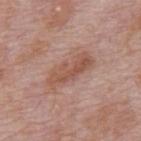No biopsy was performed on this lesion — it was imaged during a full skin examination and was not determined to be concerning. The total-body-photography lesion software estimated an automated nevus-likeness rating near 0 out of 100 and lesion-presence confidence of about 100/100. This image is a 15 mm lesion crop taken from a total-body photograph. The lesion is located on the mid back. Captured under white-light illumination. A male patient aged around 75. Longest diameter approximately 5.5 mm.